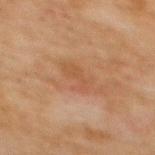<lesion>
<biopsy_status>not biopsied; imaged during a skin examination</biopsy_status>
<patient>
  <sex>female</sex>
  <age_approx>70</age_approx>
</patient>
<site>upper back</site>
<lesion_size>
  <long_diameter_mm_approx>3.5</long_diameter_mm_approx>
</lesion_size>
<image>
  <source>total-body photography crop</source>
  <field_of_view_mm>15</field_of_view_mm>
</image>
</lesion>